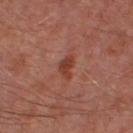biopsy status = no biopsy performed (imaged during a skin exam); tile lighting = cross-polarized; imaging modality = ~15 mm tile from a whole-body skin photo; anatomic site = the leg; patient = male, aged 63–67.